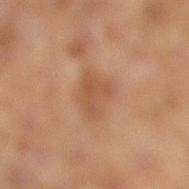The lesion was photographed on a routine skin check and not biopsied; there is no pathology result. This is a cross-polarized tile. Approximately 3.5 mm at its widest. Cropped from a whole-body photographic skin survey; the tile spans about 15 mm. Located on the leg. The patient is a female aged 58–62.